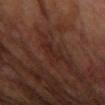workup = imaged on a skin check; not biopsied
automated lesion analysis = about 5 CIELAB-L* units darker than the surrounding skin and a normalized lesion–skin contrast near 5.5; a border-irregularity index near 5.5/10, a within-lesion color-variation index near 1.5/10, and peripheral color asymmetry of about 0.5
patient = female, aged 63 to 67
site = the right forearm
lesion size = ~3 mm (longest diameter)
imaging modality = ~15 mm crop, total-body skin-cancer survey